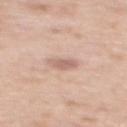The lesion-visualizer software estimated an outline eccentricity of about 0.75 (0 = round, 1 = elongated) and two-axis asymmetry of about 0.25. And it measured a border-irregularity rating of about 2/10 and a peripheral color-asymmetry measure near 0.5. The lesion is located on the back. About 2.5 mm across. Cropped from a total-body skin-imaging series; the visible field is about 15 mm. Captured under white-light illumination. A female patient, aged around 55.